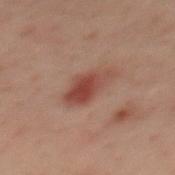notes: imaged on a skin check; not biopsied | automated metrics: an outline eccentricity of about 0.85 (0 = round, 1 = elongated) and a symmetry-axis asymmetry near 0.25; a mean CIELAB color near L≈35 a*≈20 b*≈22 and a lesion–skin lightness drop of about 8; an automated nevus-likeness rating near 95 out of 100 and lesion-presence confidence of about 100/100 | lighting: cross-polarized illumination | subject: male, aged approximately 40 | size: ≈4.5 mm | anatomic site: the mid back | image source: total-body-photography crop, ~15 mm field of view.